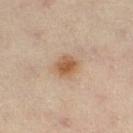Captured during whole-body skin photography for melanoma surveillance; the lesion was not biopsied.
This is a cross-polarized tile.
Cropped from a whole-body photographic skin survey; the tile spans about 15 mm.
On the leg.
A female patient aged around 55.
The lesion's longest dimension is about 2.5 mm.